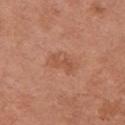Q: Is there a histopathology result?
A: catalogued during a skin exam; not biopsied
Q: How was this image acquired?
A: ~15 mm tile from a whole-body skin photo
Q: Lesion location?
A: the arm
Q: Patient demographics?
A: female, in their mid- to late 60s